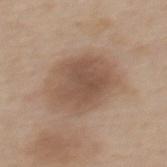Clinical impression:
The lesion was tiled from a total-body skin photograph and was not biopsied.